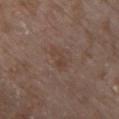Captured during whole-body skin photography for melanoma surveillance; the lesion was not biopsied. Automated tile analysis of the lesion measured a footprint of about 3.5 mm², an outline eccentricity of about 0.9 (0 = round, 1 = elongated), and a symmetry-axis asymmetry near 0.5. And it measured a nevus-likeness score of about 0/100 and a lesion-detection confidence of about 100/100. A 15 mm crop from a total-body photograph taken for skin-cancer surveillance. Imaged with white-light lighting. The lesion is on the chest. A female subject, approximately 85 years of age or older. About 3 mm across.